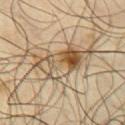follow-up = no biopsy performed (imaged during a skin exam) | acquisition = ~15 mm tile from a whole-body skin photo | subject = male, aged approximately 65 | body site = the chest.